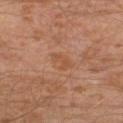No biopsy was performed on this lesion — it was imaged during a full skin examination and was not determined to be concerning.
The lesion is located on the left leg.
This is a cross-polarized tile.
A roughly 15 mm field-of-view crop from a total-body skin photograph.
About 3 mm across.
Automated image analysis of the tile measured an area of roughly 4.5 mm², an eccentricity of roughly 0.7, and a shape-asymmetry score of about 0.25 (0 = symmetric).
A male patient about 30 years old.